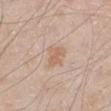Q: Was a biopsy performed?
A: no biopsy performed (imaged during a skin exam)